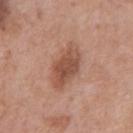{
  "biopsy_status": "not biopsied; imaged during a skin examination",
  "image": {
    "source": "total-body photography crop",
    "field_of_view_mm": 15
  },
  "patient": {
    "sex": "male",
    "age_approx": 70
  },
  "site": "chest"
}